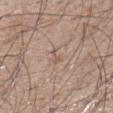Q: Is there a histopathology result?
A: catalogued during a skin exam; not biopsied
Q: What kind of image is this?
A: ~15 mm tile from a whole-body skin photo
Q: What did automated image analysis measure?
A: a mean CIELAB color near L≈56 a*≈16 b*≈25 and a lesion–skin lightness drop of about 7; lesion-presence confidence of about 50/100
Q: Lesion location?
A: the left lower leg
Q: Lesion size?
A: about 2.5 mm
Q: Patient demographics?
A: male, aged approximately 30